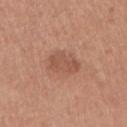Q: Was a biopsy performed?
A: total-body-photography surveillance lesion; no biopsy
Q: How was the tile lit?
A: white-light
Q: How was this image acquired?
A: 15 mm crop, total-body photography
Q: How large is the lesion?
A: about 3.5 mm
Q: What is the anatomic site?
A: the right upper arm
Q: Who is the patient?
A: female, in their mid-60s
Q: What did automated image analysis measure?
A: an area of roughly 9 mm² and two-axis asymmetry of about 0.15; a border-irregularity index near 2/10 and a color-variation rating of about 2.5/10